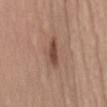Impression:
Captured during whole-body skin photography for melanoma surveillance; the lesion was not biopsied.
Image and clinical context:
The recorded lesion diameter is about 4 mm. A lesion tile, about 15 mm wide, cut from a 3D total-body photograph. A female patient aged 48–52. From the back. Imaged with white-light lighting.